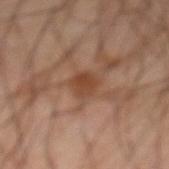<record>
<biopsy_status>not biopsied; imaged during a skin examination</biopsy_status>
<patient>
  <sex>male</sex>
  <age_approx>65</age_approx>
</patient>
<site>front of the torso</site>
<lighting>cross-polarized</lighting>
<image>
  <source>total-body photography crop</source>
  <field_of_view_mm>15</field_of_view_mm>
</image>
</record>